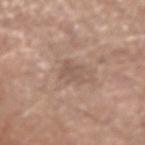notes = catalogued during a skin exam; not biopsied | site = the left forearm | acquisition = ~15 mm tile from a whole-body skin photo | subject = male, aged approximately 65.